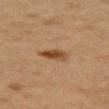Q: Was a biopsy performed?
A: no biopsy performed (imaged during a skin exam)
Q: Patient demographics?
A: female, roughly 40 years of age
Q: What is the lesion's diameter?
A: about 3.5 mm
Q: Automated lesion metrics?
A: a mean CIELAB color near L≈40 a*≈18 b*≈31, about 10 CIELAB-L* units darker than the surrounding skin, and a lesion-to-skin contrast of about 9 (normalized; higher = more distinct)
Q: What lighting was used for the tile?
A: cross-polarized
Q: What is the imaging modality?
A: ~15 mm crop, total-body skin-cancer survey
Q: Lesion location?
A: the arm